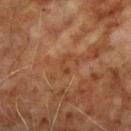Assessment:
This lesion was catalogued during total-body skin photography and was not selected for biopsy.